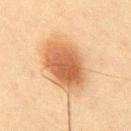biopsy status: total-body-photography surveillance lesion; no biopsy | subject: male, in their mid- to late 40s | imaging modality: total-body-photography crop, ~15 mm field of view | body site: the chest.